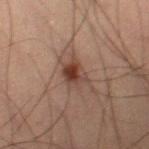Imaged during a routine full-body skin examination; the lesion was not biopsied and no histopathology is available. A region of skin cropped from a whole-body photographic capture, roughly 15 mm wide. Approximately 3.5 mm at its widest. Imaged with cross-polarized lighting. Automated tile analysis of the lesion measured a lesion area of about 7 mm², an eccentricity of roughly 0.7, and a shape-asymmetry score of about 0.3 (0 = symmetric). The software also gave a mean CIELAB color near L≈35 a*≈17 b*≈22, roughly 9 lightness units darker than nearby skin, and a lesion-to-skin contrast of about 8.5 (normalized; higher = more distinct). It also reported border irregularity of about 3.5 on a 0–10 scale, a color-variation rating of about 7.5/10, and radial color variation of about 2.5. The software also gave a nevus-likeness score of about 90/100 and a lesion-detection confidence of about 100/100. The subject is a male aged approximately 55. From the left thigh.